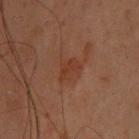Assessment:
Recorded during total-body skin imaging; not selected for excision or biopsy.
Clinical summary:
This image is a 15 mm lesion crop taken from a total-body photograph. A female patient, in their mid-50s. The lesion is located on the head or neck.